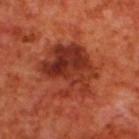Captured during whole-body skin photography for melanoma surveillance; the lesion was not biopsied. A male patient, aged 68–72. From the upper back. Captured under cross-polarized illumination. The lesion's longest dimension is about 7 mm. A lesion tile, about 15 mm wide, cut from a 3D total-body photograph.